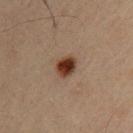This lesion was catalogued during total-body skin photography and was not selected for biopsy. The patient is a male approximately 35 years of age. The lesion is on the upper back. Cropped from a total-body skin-imaging series; the visible field is about 15 mm.